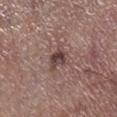Recorded during total-body skin imaging; not selected for excision or biopsy. Automated tile analysis of the lesion measured a mean CIELAB color near L≈43 a*≈17 b*≈19 and a normalized lesion–skin contrast near 8.5. The analysis additionally found border irregularity of about 3.5 on a 0–10 scale, a color-variation rating of about 5.5/10, and peripheral color asymmetry of about 2.5. The analysis additionally found a detector confidence of about 100 out of 100 that the crop contains a lesion. Imaged with white-light lighting. The lesion is on the right lower leg. A male patient aged 53–57. A roughly 15 mm field-of-view crop from a total-body skin photograph. The recorded lesion diameter is about 3 mm.